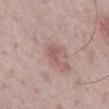- notes · imaged on a skin check; not biopsied
- body site · the right lower leg
- patient · male, aged 58 to 62
- image source · ~15 mm tile from a whole-body skin photo